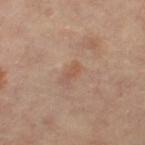<lesion>
<biopsy_status>not biopsied; imaged during a skin examination</biopsy_status>
<site>left thigh</site>
<image>
  <source>total-body photography crop</source>
  <field_of_view_mm>15</field_of_view_mm>
</image>
<lesion_size>
  <long_diameter_mm_approx>2.0</long_diameter_mm_approx>
</lesion_size>
<patient>
  <sex>female</sex>
  <age_approx>60</age_approx>
</patient>
<lighting>cross-polarized</lighting>
<automated_metrics>
  <vs_skin_darker_L>7.0</vs_skin_darker_L>
  <vs_skin_contrast_norm>5.5</vs_skin_contrast_norm>
  <color_variation_0_10>0.0</color_variation_0_10>
  <peripheral_color_asymmetry>0.0</peripheral_color_asymmetry>
  <nevus_likeness_0_100>0</nevus_likeness_0_100>
  <lesion_detection_confidence_0_100>100</lesion_detection_confidence_0_100>
</automated_metrics>
</lesion>